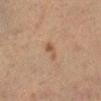No biopsy was performed on this lesion — it was imaged during a full skin examination and was not determined to be concerning. The tile uses cross-polarized illumination. A female patient aged 53 to 57. A 15 mm close-up extracted from a 3D total-body photography capture. An algorithmic analysis of the crop reported border irregularity of about 3.5 on a 0–10 scale, internal color variation of about 0.5 on a 0–10 scale, and radial color variation of about 0. The analysis additionally found a nevus-likeness score of about 10/100 and lesion-presence confidence of about 100/100. Located on the left lower leg.